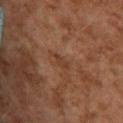The lesion was photographed on a routine skin check and not biopsied; there is no pathology result. The subject is a female about 60 years old. A roughly 15 mm field-of-view crop from a total-body skin photograph. On the upper back.